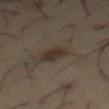Clinical summary:
Cropped from a total-body skin-imaging series; the visible field is about 15 mm. The lesion is located on the front of the torso. A male subject, about 55 years old.